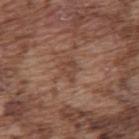Part of a total-body skin-imaging series; this lesion was reviewed on a skin check and was not flagged for biopsy. Imaged with white-light lighting. About 3 mm across. A lesion tile, about 15 mm wide, cut from a 3D total-body photograph. From the back. The subject is a male in their mid- to late 70s.